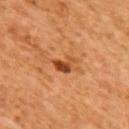Captured during whole-body skin photography for melanoma surveillance; the lesion was not biopsied. Automated image analysis of the tile measured lesion-presence confidence of about 100/100. A roughly 15 mm field-of-view crop from a total-body skin photograph. Located on the upper back. Longest diameter approximately 3 mm. A female subject, aged 63–67.